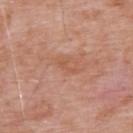  lighting: white-light
  image:
    source: total-body photography crop
    field_of_view_mm: 15
  patient:
    sex: male
    age_approx: 60
  site: upper back
  automated_metrics:
    border_irregularity_0_10: 6.5
    color_variation_0_10: 0.0
    peripheral_color_asymmetry: 0.0
  lesion_size:
    long_diameter_mm_approx: 2.5